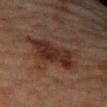Imaged during a routine full-body skin examination; the lesion was not biopsied and no histopathology is available. Automated tile analysis of the lesion measured an eccentricity of roughly 0.85 and two-axis asymmetry of about 0.35. And it measured a border-irregularity rating of about 4.5/10, a color-variation rating of about 3.5/10, and radial color variation of about 1.5. And it measured an automated nevus-likeness rating near 35 out of 100. A lesion tile, about 15 mm wide, cut from a 3D total-body photograph. Captured under cross-polarized illumination. Approximately 6.5 mm at its widest. The patient is a female aged around 80. From the right forearm.